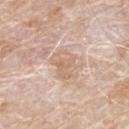Q: What is the imaging modality?
A: ~15 mm crop, total-body skin-cancer survey
Q: What lighting was used for the tile?
A: white-light illumination
Q: What did automated image analysis measure?
A: a nevus-likeness score of about 0/100 and a detector confidence of about 90 out of 100 that the crop contains a lesion
Q: What is the anatomic site?
A: the mid back
Q: Patient demographics?
A: male, aged 78–82